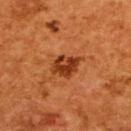Clinical impression:
Imaged during a routine full-body skin examination; the lesion was not biopsied and no histopathology is available.
Image and clinical context:
A female subject approximately 50 years of age. The lesion is on the back. A close-up tile cropped from a whole-body skin photograph, about 15 mm across.